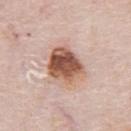workup: imaged on a skin check; not biopsied
diameter: ~5 mm (longest diameter)
body site: the chest
imaging modality: 15 mm crop, total-body photography
tile lighting: white-light illumination
patient: male, aged around 80